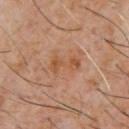{
  "biopsy_status": "not biopsied; imaged during a skin examination",
  "site": "chest",
  "image": {
    "source": "total-body photography crop",
    "field_of_view_mm": 15
  },
  "patient": {
    "sex": "male",
    "age_approx": 60
  }
}